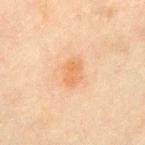<record>
<biopsy_status>not biopsied; imaged during a skin examination</biopsy_status>
<image>
  <source>total-body photography crop</source>
  <field_of_view_mm>15</field_of_view_mm>
</image>
<site>chest</site>
<patient>
  <sex>female</sex>
  <age_approx>50</age_approx>
</patient>
</record>